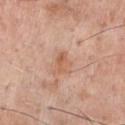Clinical impression:
Part of a total-body skin-imaging series; this lesion was reviewed on a skin check and was not flagged for biopsy.
Context:
On the chest. A male patient, roughly 60 years of age. A 15 mm crop from a total-body photograph taken for skin-cancer surveillance. The tile uses white-light illumination. Automated image analysis of the tile measured a footprint of about 4 mm², an eccentricity of roughly 0.75, and two-axis asymmetry of about 0.25. Longest diameter approximately 2.5 mm.